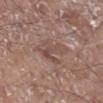Assessment:
Part of a total-body skin-imaging series; this lesion was reviewed on a skin check and was not flagged for biopsy.
Background:
Cropped from a whole-body photographic skin survey; the tile spans about 15 mm. Imaged with white-light lighting. The lesion is located on the right lower leg. The patient is a male aged around 70. Approximately 4.5 mm at its widest.